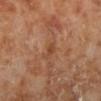{"image": {"source": "total-body photography crop", "field_of_view_mm": 15}, "automated_metrics": {"area_mm2_approx": 2.5, "eccentricity": 0.85, "shape_asymmetry": 0.4, "border_irregularity_0_10": 4.0, "color_variation_0_10": 1.0, "peripheral_color_asymmetry": 0.5, "lesion_detection_confidence_0_100": 100}, "site": "leg", "patient": {"sex": "male", "age_approx": 70}, "lesion_size": {"long_diameter_mm_approx": 2.5}}